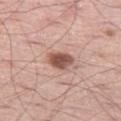Captured during whole-body skin photography for melanoma surveillance; the lesion was not biopsied. Imaged with white-light lighting. A male patient, in their 60s. A lesion tile, about 15 mm wide, cut from a 3D total-body photograph. Automated image analysis of the tile measured an area of roughly 6.5 mm². The lesion's longest dimension is about 3.5 mm. Located on the left thigh.